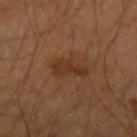| feature | finding |
|---|---|
| workup | no biopsy performed (imaged during a skin exam) |
| acquisition | 15 mm crop, total-body photography |
| patient | male, in their 50s |
| location | the left arm |
| lighting | cross-polarized |